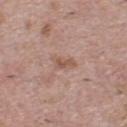The lesion is located on the chest. A lesion tile, about 15 mm wide, cut from a 3D total-body photograph. This is a white-light tile. The recorded lesion diameter is about 3 mm. The patient is a male aged approximately 40.